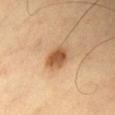<lesion>
  <patient>
    <sex>male</sex>
    <age_approx>50</age_approx>
  </patient>
  <image>
    <source>total-body photography crop</source>
    <field_of_view_mm>15</field_of_view_mm>
  </image>
  <automated_metrics>
    <vs_skin_darker_L>14.0</vs_skin_darker_L>
    <vs_skin_contrast_norm>9.0</vs_skin_contrast_norm>
    <nevus_likeness_0_100>95</nevus_likeness_0_100>
  </automated_metrics>
  <lighting>cross-polarized</lighting>
  <site>chest</site>
  <lesion_size>
    <long_diameter_mm_approx>3.0</long_diameter_mm_approx>
  </lesion_size>
</lesion>